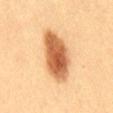| feature | finding |
|---|---|
| follow-up | no biopsy performed (imaged during a skin exam) |
| acquisition | ~15 mm crop, total-body skin-cancer survey |
| patient | female, aged 28–32 |
| anatomic site | the mid back |